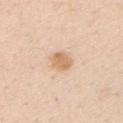notes: no biopsy performed (imaged during a skin exam)
patient: male, aged around 30
TBP lesion metrics: roughly 11 lightness units darker than nearby skin and a lesion-to-skin contrast of about 7.5 (normalized; higher = more distinct); a border-irregularity index near 1.5/10, a within-lesion color-variation index near 2/10, and a peripheral color-asymmetry measure near 0.5
image source: total-body-photography crop, ~15 mm field of view
diameter: ~2.5 mm (longest diameter)
anatomic site: the arm